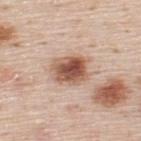biopsy status=total-body-photography surveillance lesion; no biopsy
tile lighting=white-light illumination
location=the upper back
lesion diameter=≈4.5 mm
subject=male, roughly 45 years of age
TBP lesion metrics=a shape eccentricity near 0.65; a lesion color around L≈56 a*≈21 b*≈29 in CIELAB and a normalized lesion–skin contrast near 10.5; a border-irregularity index near 2/10 and a within-lesion color-variation index near 8/10
imaging modality=15 mm crop, total-body photography